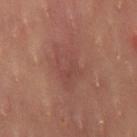{
  "biopsy_status": "not biopsied; imaged during a skin examination",
  "patient": {
    "sex": "female",
    "age_approx": 35
  },
  "automated_metrics": {
    "eccentricity": 0.85
  },
  "image": {
    "source": "total-body photography crop",
    "field_of_view_mm": 15
  },
  "site": "leg"
}